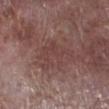The lesion was tiled from a total-body skin photograph and was not biopsied. Captured under white-light illumination. A 15 mm crop from a total-body photograph taken for skin-cancer surveillance. A male patient, aged 78–82. On the right lower leg. The total-body-photography lesion software estimated a lesion area of about 11 mm², an outline eccentricity of about 0.65 (0 = round, 1 = elongated), and a symmetry-axis asymmetry near 0.5. The software also gave a border-irregularity index near 9/10, a color-variation rating of about 2/10, and peripheral color asymmetry of about 0.5. And it measured a detector confidence of about 95 out of 100 that the crop contains a lesion.